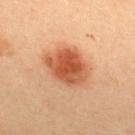follow-up = imaged on a skin check; not biopsied
automated metrics = an automated nevus-likeness rating near 100 out of 100 and a lesion-detection confidence of about 100/100
anatomic site = the upper back
patient = female, aged approximately 35
lighting = cross-polarized
diameter = ~5.5 mm (longest diameter)
imaging modality = total-body-photography crop, ~15 mm field of view